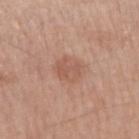Q: Lesion location?
A: the right upper arm
Q: What lighting was used for the tile?
A: white-light
Q: What did automated image analysis measure?
A: a border-irregularity index near 2/10, internal color variation of about 2.5 on a 0–10 scale, and a peripheral color-asymmetry measure near 1
Q: What is the imaging modality?
A: ~15 mm crop, total-body skin-cancer survey
Q: Patient demographics?
A: male, approximately 60 years of age
Q: How large is the lesion?
A: about 3 mm